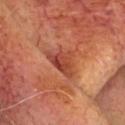biopsy status = imaged on a skin check; not biopsied
imaging modality = 15 mm crop, total-body photography
lighting = cross-polarized
patient = aged approximately 65
automated lesion analysis = a color-variation rating of about 5.5/10 and a peripheral color-asymmetry measure near 1.5
lesion diameter = about 4 mm
anatomic site = the head or neck